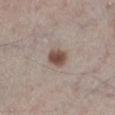| field | value |
|---|---|
| biopsy status | no biopsy performed (imaged during a skin exam) |
| TBP lesion metrics | a footprint of about 5.5 mm² and a symmetry-axis asymmetry near 0.15 |
| size | ≈2.5 mm |
| body site | the leg |
| imaging modality | 15 mm crop, total-body photography |
| subject | male, roughly 60 years of age |
| lighting | white-light illumination |